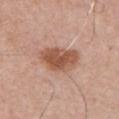{
  "biopsy_status": "not biopsied; imaged during a skin examination",
  "site": "chest",
  "image": {
    "source": "total-body photography crop",
    "field_of_view_mm": 15
  },
  "patient": {
    "sex": "male",
    "age_approx": 70
  }
}